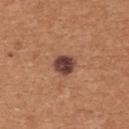| key | value |
|---|---|
| follow-up | imaged on a skin check; not biopsied |
| subject | female, aged around 35 |
| location | the upper back |
| lesion size | about 2.5 mm |
| tile lighting | white-light |
| acquisition | ~15 mm tile from a whole-body skin photo |